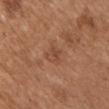Q: Was a biopsy performed?
A: catalogued during a skin exam; not biopsied
Q: What lighting was used for the tile?
A: white-light illumination
Q: Lesion location?
A: the front of the torso
Q: What is the lesion's diameter?
A: ~3 mm (longest diameter)
Q: Automated lesion metrics?
A: an area of roughly 4.5 mm², an eccentricity of roughly 0.6, and two-axis asymmetry of about 0.3; roughly 7 lightness units darker than nearby skin and a normalized lesion–skin contrast near 5.5
Q: Who is the patient?
A: male, aged around 65
Q: What is the imaging modality?
A: ~15 mm crop, total-body skin-cancer survey